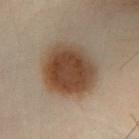Part of a total-body skin-imaging series; this lesion was reviewed on a skin check and was not flagged for biopsy. A lesion tile, about 15 mm wide, cut from a 3D total-body photograph. The lesion is located on the left lower leg. The lesion's longest dimension is about 6.5 mm. Imaged with cross-polarized lighting. A male subject approximately 50 years of age.